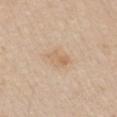Captured during whole-body skin photography for melanoma surveillance; the lesion was not biopsied. A 15 mm crop from a total-body photograph taken for skin-cancer surveillance. On the front of the torso. The subject is a male roughly 65 years of age.